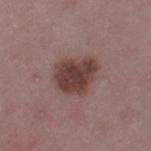Imaged with white-light lighting.
Cropped from a whole-body photographic skin survey; the tile spans about 15 mm.
Located on the leg.
A female patient in their mid-40s.